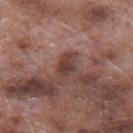The lesion was tiled from a total-body skin photograph and was not biopsied. A lesion tile, about 15 mm wide, cut from a 3D total-body photograph. The lesion is located on the mid back. This is a white-light tile. The lesion's longest dimension is about 3 mm. A male patient aged 68–72.